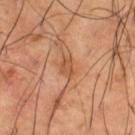Q: What is the lesion's diameter?
A: ≈2.5 mm
Q: Patient demographics?
A: male, aged around 65
Q: What is the imaging modality?
A: ~15 mm tile from a whole-body skin photo
Q: Illumination type?
A: cross-polarized illumination
Q: What is the anatomic site?
A: the right thigh